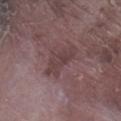The lesion was tiled from a total-body skin photograph and was not biopsied. The subject is a male aged 73 to 77. This image is a 15 mm lesion crop taken from a total-body photograph. An algorithmic analysis of the crop reported a lesion color around L≈39 a*≈18 b*≈16 in CIELAB and a lesion–skin lightness drop of about 7. And it measured a classifier nevus-likeness of about 0/100. Imaged with white-light lighting. Approximately 4.5 mm at its widest. On the leg.